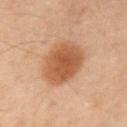{"biopsy_status": "not biopsied; imaged during a skin examination", "lighting": "cross-polarized", "patient": {"sex": "male", "age_approx": 50}, "automated_metrics": {"border_irregularity_0_10": 1.0, "nevus_likeness_0_100": 100}, "image": {"source": "total-body photography crop", "field_of_view_mm": 15}, "lesion_size": {"long_diameter_mm_approx": 5.0}, "site": "abdomen"}